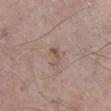Q: Was a biopsy performed?
A: total-body-photography surveillance lesion; no biopsy
Q: Automated lesion metrics?
A: an eccentricity of roughly 0.9; a border-irregularity index near 5.5/10, a within-lesion color-variation index near 0/10, and peripheral color asymmetry of about 0
Q: What are the patient's age and sex?
A: male, about 70 years old
Q: How was this image acquired?
A: ~15 mm tile from a whole-body skin photo
Q: Lesion location?
A: the leg
Q: How large is the lesion?
A: about 3 mm
Q: How was the tile lit?
A: white-light